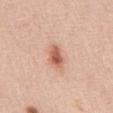The total-body-photography lesion software estimated an area of roughly 5.5 mm², an outline eccentricity of about 0.8 (0 = round, 1 = elongated), and two-axis asymmetry of about 0.25. And it measured a border-irregularity rating of about 2.5/10, a within-lesion color-variation index near 4.5/10, and radial color variation of about 1.5. Captured under white-light illumination. A region of skin cropped from a whole-body photographic capture, roughly 15 mm wide. Measured at roughly 3.5 mm in maximum diameter. The patient is a male about 50 years old. The lesion is on the front of the torso.